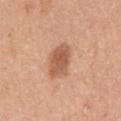The lesion-visualizer software estimated an eccentricity of roughly 0.6. It also reported a mean CIELAB color near L≈57 a*≈23 b*≈33 and a lesion-to-skin contrast of about 7.5 (normalized; higher = more distinct). Cropped from a total-body skin-imaging series; the visible field is about 15 mm. The lesion is located on the right upper arm. About 3.5 mm across. The patient is a female roughly 35 years of age. Imaged with white-light lighting.